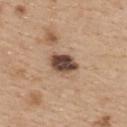biopsy status: total-body-photography surveillance lesion; no biopsy
illumination: white-light illumination
acquisition: ~15 mm tile from a whole-body skin photo
automated lesion analysis: border irregularity of about 2 on a 0–10 scale
anatomic site: the upper back
subject: female, in their mid- to late 40s
lesion size: ~3.5 mm (longest diameter)